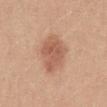{"biopsy_status": "not biopsied; imaged during a skin examination", "patient": {"sex": "female", "age_approx": 30}, "site": "abdomen", "image": {"source": "total-body photography crop", "field_of_view_mm": 15}}